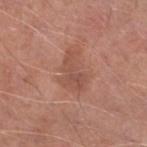Assessment: Recorded during total-body skin imaging; not selected for excision or biopsy. Background: This is a white-light tile. A 15 mm close-up extracted from a 3D total-body photography capture. Automated tile analysis of the lesion measured a lesion area of about 8.5 mm² and a shape eccentricity near 0.8. The analysis additionally found border irregularity of about 6 on a 0–10 scale and a color-variation rating of about 3/10. The software also gave a classifier nevus-likeness of about 0/100 and lesion-presence confidence of about 100/100. The subject is a male aged 63 to 67. On the right lower leg. The lesion's longest dimension is about 4.5 mm.